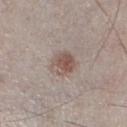Impression:
Imaged during a routine full-body skin examination; the lesion was not biopsied and no histopathology is available.
Image and clinical context:
From the left lower leg. An algorithmic analysis of the crop reported a footprint of about 7.5 mm², a shape eccentricity near 0.5, and a symmetry-axis asymmetry near 0.1. And it measured an average lesion color of about L≈53 a*≈15 b*≈21 (CIELAB), roughly 10 lightness units darker than nearby skin, and a normalized lesion–skin contrast near 7. The subject is a male aged 58–62. The recorded lesion diameter is about 3.5 mm. A close-up tile cropped from a whole-body skin photograph, about 15 mm across. Imaged with white-light lighting.